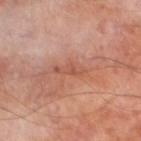follow-up — total-body-photography surveillance lesion; no biopsy
patient — male, approximately 70 years of age
acquisition — ~15 mm crop, total-body skin-cancer survey
site — the left lower leg